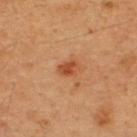Case summary:
- subject: male, about 50 years old
- site: the upper back
- illumination: cross-polarized
- imaging modality: 15 mm crop, total-body photography